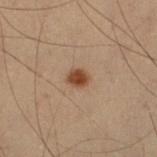workup = imaged on a skin check; not biopsied
patient = male, aged approximately 55
anatomic site = the leg
imaging modality = 15 mm crop, total-body photography